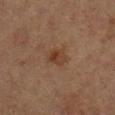| feature | finding |
|---|---|
| biopsy status | catalogued during a skin exam; not biopsied |
| lesion diameter | ~2.5 mm (longest diameter) |
| image | ~15 mm tile from a whole-body skin photo |
| illumination | cross-polarized |
| patient | male, in their mid-80s or older |
| location | the right lower leg |